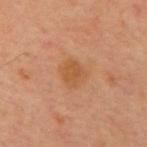Impression:
No biopsy was performed on this lesion — it was imaged during a full skin examination and was not determined to be concerning.
Background:
Imaged with cross-polarized lighting. A 15 mm close-up tile from a total-body photography series done for melanoma screening. A male subject, roughly 60 years of age. The lesion is on the chest. Measured at roughly 3 mm in maximum diameter.